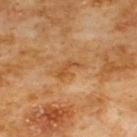biopsy status = no biopsy performed (imaged during a skin exam) | site = the front of the torso | patient = male, aged 58–62 | imaging modality = ~15 mm crop, total-body skin-cancer survey.